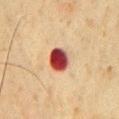- biopsy status — total-body-photography surveillance lesion; no biopsy
- image — ~15 mm crop, total-body skin-cancer survey
- site — the chest
- tile lighting — cross-polarized
- subject — male, roughly 60 years of age
- lesion diameter — ~3 mm (longest diameter)